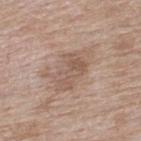Assessment: No biopsy was performed on this lesion — it was imaged during a full skin examination and was not determined to be concerning. Acquisition and patient details: Cropped from a total-body skin-imaging series; the visible field is about 15 mm. Automated tile analysis of the lesion measured border irregularity of about 4.5 on a 0–10 scale, a within-lesion color-variation index near 3.5/10, and a peripheral color-asymmetry measure near 1.5. It also reported lesion-presence confidence of about 100/100. A female patient in their mid- to late 70s. The lesion is located on the upper back. The recorded lesion diameter is about 5.5 mm.